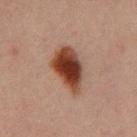Q: Is there a histopathology result?
A: imaged on a skin check; not biopsied
Q: How was this image acquired?
A: 15 mm crop, total-body photography
Q: Patient demographics?
A: male, in their 30s
Q: What is the lesion's diameter?
A: ~5.5 mm (longest diameter)
Q: Lesion location?
A: the mid back
Q: How was the tile lit?
A: cross-polarized illumination